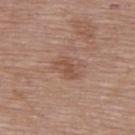Assessment: This lesion was catalogued during total-body skin photography and was not selected for biopsy. Context: A close-up tile cropped from a whole-body skin photograph, about 15 mm across. Imaged with white-light lighting. The lesion-visualizer software estimated an average lesion color of about L≈51 a*≈20 b*≈28 (CIELAB), roughly 7 lightness units darker than nearby skin, and a lesion-to-skin contrast of about 5.5 (normalized; higher = more distinct). From the upper back. Longest diameter approximately 3.5 mm. A female subject roughly 50 years of age.